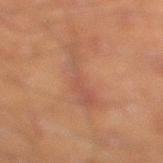Part of a total-body skin-imaging series; this lesion was reviewed on a skin check and was not flagged for biopsy. A male subject, about 45 years old. Measured at roughly 6.5 mm in maximum diameter. The tile uses cross-polarized illumination. Cropped from a total-body skin-imaging series; the visible field is about 15 mm. The lesion is located on the right lower leg.